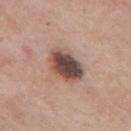Captured during whole-body skin photography for melanoma surveillance; the lesion was not biopsied. Measured at roughly 4.5 mm in maximum diameter. An algorithmic analysis of the crop reported an average lesion color of about L≈46 a*≈18 b*≈22 (CIELAB) and a lesion-to-skin contrast of about 12.5 (normalized; higher = more distinct). A female subject, aged approximately 55. Imaged with white-light lighting. A region of skin cropped from a whole-body photographic capture, roughly 15 mm wide. The lesion is located on the left thigh.